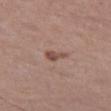This lesion was catalogued during total-body skin photography and was not selected for biopsy. A 15 mm close-up extracted from a 3D total-body photography capture. The lesion is located on the left thigh. A male subject, aged around 70.